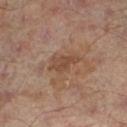Impression:
The lesion was tiled from a total-body skin photograph and was not biopsied.
Context:
Automated image analysis of the tile measured an area of roughly 5 mm², a shape eccentricity near 0.85, and a symmetry-axis asymmetry near 0.35. Captured under cross-polarized illumination. The lesion's longest dimension is about 4 mm. A region of skin cropped from a whole-body photographic capture, roughly 15 mm wide. From the left lower leg. A male patient, aged approximately 65.